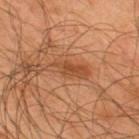| key | value |
|---|---|
| notes | imaged on a skin check; not biopsied |
| subject | male, in their mid-40s |
| anatomic site | the back |
| tile lighting | cross-polarized |
| size | ~5 mm (longest diameter) |
| imaging modality | 15 mm crop, total-body photography |